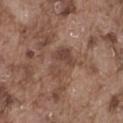{
  "site": "abdomen",
  "automated_metrics": {
    "border_irregularity_0_10": 5.5,
    "color_variation_0_10": 3.0,
    "peripheral_color_asymmetry": 1.0
  },
  "lighting": "white-light",
  "patient": {
    "sex": "male",
    "age_approx": 75
  },
  "lesion_size": {
    "long_diameter_mm_approx": 4.0
  },
  "image": {
    "source": "total-body photography crop",
    "field_of_view_mm": 15
  }
}